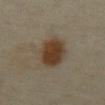The lesion was photographed on a routine skin check and not biopsied; there is no pathology result.
This image is a 15 mm lesion crop taken from a total-body photograph.
From the abdomen.
A female subject aged 33–37.